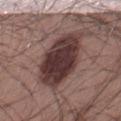Captured during whole-body skin photography for melanoma surveillance; the lesion was not biopsied.
A 15 mm close-up extracted from a 3D total-body photography capture.
Located on the right thigh.
Measured at roughly 8 mm in maximum diameter.
Captured under white-light illumination.
A male subject, aged approximately 55.